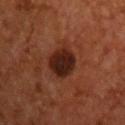{
  "biopsy_status": "not biopsied; imaged during a skin examination",
  "lighting": "cross-polarized",
  "image": {
    "source": "total-body photography crop",
    "field_of_view_mm": 15
  },
  "patient": {
    "sex": "male",
    "age_approx": 55
  },
  "site": "chest",
  "lesion_size": {
    "long_diameter_mm_approx": 3.5
  }
}